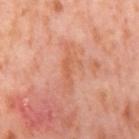workup: imaged on a skin check; not biopsied | image source: ~15 mm tile from a whole-body skin photo | lesion size: ≈5.5 mm | subject: female, approximately 55 years of age | TBP lesion metrics: an outline eccentricity of about 0.95 (0 = round, 1 = elongated) and a shape-asymmetry score of about 0.45 (0 = symmetric); a border-irregularity index near 7/10, a color-variation rating of about 1.5/10, and peripheral color asymmetry of about 0.5 | anatomic site: the right thigh.